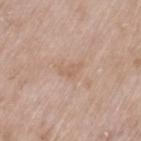Case summary:
- workup — catalogued during a skin exam; not biopsied
- patient — female, aged 73 to 77
- location — the left thigh
- automated lesion analysis — an outline eccentricity of about 0.85 (0 = round, 1 = elongated) and a symmetry-axis asymmetry near 0.45; about 6 CIELAB-L* units darker than the surrounding skin; a nevus-likeness score of about 0/100 and lesion-presence confidence of about 100/100
- image — total-body-photography crop, ~15 mm field of view
- lighting — white-light illumination